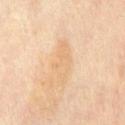Clinical impression: Recorded during total-body skin imaging; not selected for excision or biopsy. Acquisition and patient details: On the chest. This is a cross-polarized tile. The patient is a female in their mid-40s. The lesion's longest dimension is about 5 mm. A lesion tile, about 15 mm wide, cut from a 3D total-body photograph.